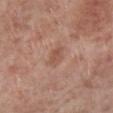biopsy status: catalogued during a skin exam; not biopsied